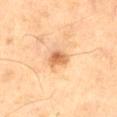Case summary:
* notes: no biopsy performed (imaged during a skin exam)